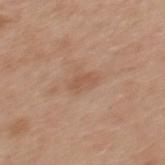Captured during whole-body skin photography for melanoma surveillance; the lesion was not biopsied.